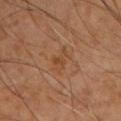biopsy status = no biopsy performed (imaged during a skin exam)
subject = male, about 60 years old
imaging modality = 15 mm crop, total-body photography
diameter = ≈3.5 mm
body site = the chest
lighting = cross-polarized illumination